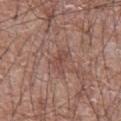Impression:
Recorded during total-body skin imaging; not selected for excision or biopsy.
Acquisition and patient details:
Located on the abdomen. A male subject aged approximately 75. The total-body-photography lesion software estimated an average lesion color of about L≈47 a*≈21 b*≈23 (CIELAB), about 7 CIELAB-L* units darker than the surrounding skin, and a normalized lesion–skin contrast near 5.5. The analysis additionally found a border-irregularity rating of about 4/10 and a within-lesion color-variation index near 2/10. Longest diameter approximately 3 mm. A region of skin cropped from a whole-body photographic capture, roughly 15 mm wide.